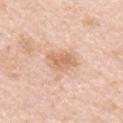follow-up: total-body-photography surveillance lesion; no biopsy | diameter: ~4 mm (longest diameter) | patient: female, aged around 45 | body site: the right upper arm | illumination: white-light illumination | automated lesion analysis: a border-irregularity index near 3/10 and a color-variation rating of about 2.5/10 | acquisition: 15 mm crop, total-body photography.